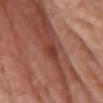Impression: This lesion was catalogued during total-body skin photography and was not selected for biopsy. Acquisition and patient details: A 15 mm close-up extracted from a 3D total-body photography capture. The total-body-photography lesion software estimated an average lesion color of about L≈40 a*≈27 b*≈28 (CIELAB), a lesion–skin lightness drop of about 9, and a lesion-to-skin contrast of about 7 (normalized; higher = more distinct). This is a white-light tile. A female patient, roughly 75 years of age. Approximately 2.5 mm at its widest. Located on the front of the torso.